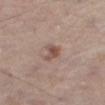Q: Was this lesion biopsied?
A: catalogued during a skin exam; not biopsied
Q: What is the imaging modality?
A: total-body-photography crop, ~15 mm field of view
Q: Patient demographics?
A: male, aged 73–77
Q: Lesion location?
A: the right thigh